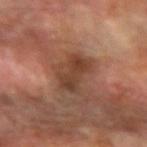Recorded during total-body skin imaging; not selected for excision or biopsy. A lesion tile, about 15 mm wide, cut from a 3D total-body photograph. The lesion is on the left forearm. A male subject, aged around 70. This is a cross-polarized tile. Measured at roughly 4.5 mm in maximum diameter.